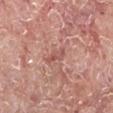Clinical impression: Recorded during total-body skin imaging; not selected for excision or biopsy. Context: The tile uses white-light illumination. A male subject approximately 65 years of age. Longest diameter approximately 3 mm. Located on the left lower leg. Automated image analysis of the tile measured a footprint of about 2.5 mm², an outline eccentricity of about 0.95 (0 = round, 1 = elongated), and two-axis asymmetry of about 0.45. A 15 mm close-up extracted from a 3D total-body photography capture.